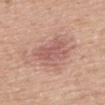| field | value |
|---|---|
| patient | male, aged around 80 |
| automated metrics | a lesion area of about 15 mm² and a symmetry-axis asymmetry near 0.25; a mean CIELAB color near L≈58 a*≈23 b*≈25, about 8 CIELAB-L* units darker than the surrounding skin, and a normalized lesion–skin contrast near 6; internal color variation of about 3 on a 0–10 scale and radial color variation of about 1 |
| image | 15 mm crop, total-body photography |
| anatomic site | the upper back |
| tile lighting | white-light |